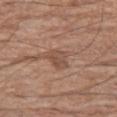Findings:
* workup — no biopsy performed (imaged during a skin exam)
* image source — ~15 mm crop, total-body skin-cancer survey
* diameter — about 2.5 mm
* illumination — white-light illumination
* anatomic site — the left thigh
* patient — male, about 65 years old